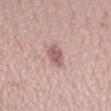<lesion>
  <biopsy_status>not biopsied; imaged during a skin examination</biopsy_status>
  <site>right forearm</site>
  <image>
    <source>total-body photography crop</source>
    <field_of_view_mm>15</field_of_view_mm>
  </image>
  <patient>
    <sex>female</sex>
    <age_approx>35</age_approx>
  </patient>
  <automated_metrics>
    <border_irregularity_0_10>2.0</border_irregularity_0_10>
    <color_variation_0_10>2.0</color_variation_0_10>
    <peripheral_color_asymmetry>0.5</peripheral_color_asymmetry>
    <nevus_likeness_0_100>35</nevus_likeness_0_100>
    <lesion_detection_confidence_0_100>100</lesion_detection_confidence_0_100>
  </automated_metrics>
  <lighting>white-light</lighting>
</lesion>